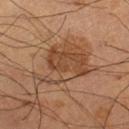| field | value |
|---|---|
| follow-up | imaged on a skin check; not biopsied |
| anatomic site | the right lower leg |
| size | ≈7 mm |
| acquisition | total-body-photography crop, ~15 mm field of view |
| patient | male, aged around 60 |